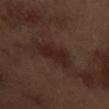Findings:
* follow-up — catalogued during a skin exam; not biopsied
* patient — male, roughly 70 years of age
* automated metrics — a footprint of about 9 mm², an outline eccentricity of about 0.85 (0 = round, 1 = elongated), and two-axis asymmetry of about 0.3; a mean CIELAB color near L≈23 a*≈17 b*≈21 and roughly 6 lightness units darker than nearby skin; a border-irregularity index near 3.5/10 and internal color variation of about 2.5 on a 0–10 scale; an automated nevus-likeness rating near 20 out of 100 and a lesion-detection confidence of about 100/100
* lighting — white-light illumination
* body site — the arm
* size — ~4.5 mm (longest diameter)
* imaging modality — ~15 mm crop, total-body skin-cancer survey